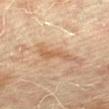No biopsy was performed on this lesion — it was imaged during a full skin examination and was not determined to be concerning.